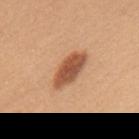No biopsy was performed on this lesion — it was imaged during a full skin examination and was not determined to be concerning.